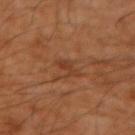Imaged during a routine full-body skin examination; the lesion was not biopsied and no histopathology is available. Cropped from a total-body skin-imaging series; the visible field is about 15 mm. The lesion is on the right forearm. A male subject in their 60s. Imaged with cross-polarized lighting. Automated image analysis of the tile measured a lesion area of about 3.5 mm² and a shape-asymmetry score of about 0.4 (0 = symmetric). The analysis additionally found an average lesion color of about L≈34 a*≈21 b*≈30 (CIELAB) and a lesion-to-skin contrast of about 5.5 (normalized; higher = more distinct).